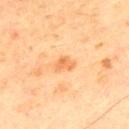<record>
  <biopsy_status>not biopsied; imaged during a skin examination</biopsy_status>
  <site>chest</site>
  <image>
    <source>total-body photography crop</source>
    <field_of_view_mm>15</field_of_view_mm>
  </image>
  <patient>
    <sex>male</sex>
    <age_approx>70</age_approx>
  </patient>
  <automated_metrics>
    <area_mm2_approx>4.0</area_mm2_approx>
    <eccentricity>0.7</eccentricity>
    <shape_asymmetry>0.3</shape_asymmetry>
    <nevus_likeness_0_100>0</nevus_likeness_0_100>
    <lesion_detection_confidence_0_100>100</lesion_detection_confidence_0_100>
  </automated_metrics>
  <lesion_size>
    <long_diameter_mm_approx>2.5</long_diameter_mm_approx>
  </lesion_size>
  <lighting>cross-polarized</lighting>
</record>